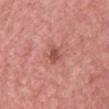* workup · imaged on a skin check; not biopsied
* patient · female, aged 38 to 42
* body site · the chest
* automated lesion analysis · an eccentricity of roughly 0.7 and a shape-asymmetry score of about 0.4 (0 = symmetric); a lesion color around L≈51 a*≈28 b*≈28 in CIELAB and a lesion–skin lightness drop of about 11
* acquisition · ~15 mm crop, total-body skin-cancer survey
* illumination · white-light illumination